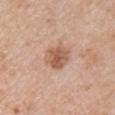notes — no biopsy performed (imaged during a skin exam)
anatomic site — the right upper arm
image — total-body-photography crop, ~15 mm field of view
subject — female, approximately 70 years of age
diameter — ≈3.5 mm